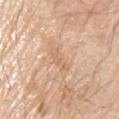patient:
  sex: male
  age_approx: 80
site: chest
image:
  source: total-body photography crop
  field_of_view_mm: 15